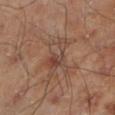Impression: The lesion was tiled from a total-body skin photograph and was not biopsied. Clinical summary: A male patient, aged 68–72. The lesion is located on the left lower leg. Approximately 5 mm at its widest. Imaged with cross-polarized lighting. A 15 mm crop from a total-body photograph taken for skin-cancer surveillance. The total-body-photography lesion software estimated a lesion–skin lightness drop of about 6 and a normalized lesion–skin contrast near 5.5. The software also gave lesion-presence confidence of about 100/100.